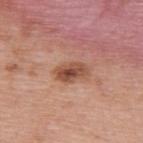Case summary:
– biopsy status — total-body-photography surveillance lesion; no biopsy
– image-analysis metrics — a lesion color around L≈50 a*≈24 b*≈30 in CIELAB, about 12 CIELAB-L* units darker than the surrounding skin, and a normalized border contrast of about 8.5; a nevus-likeness score of about 40/100
– illumination — white-light illumination
– image — ~15 mm tile from a whole-body skin photo
– body site — the upper back
– subject — female, about 40 years old
– size — ≈4 mm